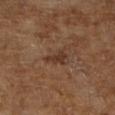Part of a total-body skin-imaging series; this lesion was reviewed on a skin check and was not flagged for biopsy. A male subject, aged 63 to 67. About 2.5 mm across. Captured under cross-polarized illumination. A region of skin cropped from a whole-body photographic capture, roughly 15 mm wide.